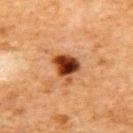  biopsy_status: not biopsied; imaged during a skin examination
  image:
    source: total-body photography crop
    field_of_view_mm: 15
  patient:
    sex: female
    age_approx: 60
  site: upper back
  lighting: cross-polarized
  lesion_size:
    long_diameter_mm_approx: 3.5
  automated_metrics:
    cielab_L: 34
    cielab_a: 24
    cielab_b: 32
    vs_skin_darker_L: 18.0
    vs_skin_contrast_norm: 14.5
    nevus_likeness_0_100: 100
    lesion_detection_confidence_0_100: 100